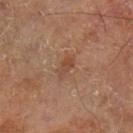Q: Was a biopsy performed?
A: catalogued during a skin exam; not biopsied